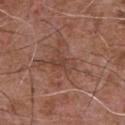Notes:
* biopsy status · total-body-photography surveillance lesion; no biopsy
* image · 15 mm crop, total-body photography
* lesion size · ~3.5 mm (longest diameter)
* patient · male, in their mid- to late 70s
* location · the chest
* tile lighting · white-light